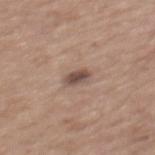Captured during whole-body skin photography for melanoma surveillance; the lesion was not biopsied. A female patient, aged around 65. This is a white-light tile. The total-body-photography lesion software estimated an area of roughly 4 mm², an eccentricity of roughly 0.85, and a shape-asymmetry score of about 0.2 (0 = symmetric). It also reported a classifier nevus-likeness of about 25/100 and a detector confidence of about 100 out of 100 that the crop contains a lesion. A roughly 15 mm field-of-view crop from a total-body skin photograph. The lesion is on the mid back. The recorded lesion diameter is about 3 mm.